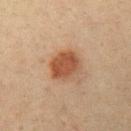Q: Was this lesion biopsied?
A: total-body-photography surveillance lesion; no biopsy
Q: How large is the lesion?
A: about 4 mm
Q: What kind of image is this?
A: 15 mm crop, total-body photography
Q: Who is the patient?
A: female, aged approximately 40
Q: Lesion location?
A: the left upper arm